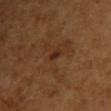Assessment: The lesion was tiled from a total-body skin photograph and was not biopsied. Clinical summary: A 15 mm close-up tile from a total-body photography series done for melanoma screening. From the upper back. An algorithmic analysis of the crop reported a footprint of about 1.5 mm², an outline eccentricity of about 0.85 (0 = round, 1 = elongated), and a symmetry-axis asymmetry near 0.4. And it measured a classifier nevus-likeness of about 0/100 and a lesion-detection confidence of about 100/100. The patient is a female aged approximately 55. This is a cross-polarized tile.